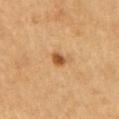This lesion was catalogued during total-body skin photography and was not selected for biopsy.
Longest diameter approximately 2 mm.
The lesion is located on the right upper arm.
A male subject, in their 70s.
An algorithmic analysis of the crop reported an area of roughly 3.5 mm² and an eccentricity of roughly 0.55. The analysis additionally found a lesion color around L≈54 a*≈23 b*≈41 in CIELAB. The software also gave a classifier nevus-likeness of about 95/100 and lesion-presence confidence of about 100/100.
A region of skin cropped from a whole-body photographic capture, roughly 15 mm wide.
The tile uses cross-polarized illumination.